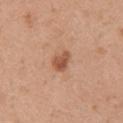The lesion was tiled from a total-body skin photograph and was not biopsied.
An algorithmic analysis of the crop reported a mean CIELAB color near L≈54 a*≈23 b*≈33 and a normalized border contrast of about 8. The analysis additionally found a border-irregularity index near 2.5/10, internal color variation of about 3.5 on a 0–10 scale, and radial color variation of about 1. It also reported lesion-presence confidence of about 100/100.
The lesion is located on the left upper arm.
Imaged with white-light lighting.
A close-up tile cropped from a whole-body skin photograph, about 15 mm across.
A female subject, approximately 40 years of age.
The recorded lesion diameter is about 3 mm.